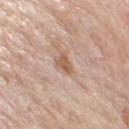The lesion was tiled from a total-body skin photograph and was not biopsied. Automated tile analysis of the lesion measured a mean CIELAB color near L≈57 a*≈20 b*≈30, roughly 10 lightness units darker than nearby skin, and a lesion-to-skin contrast of about 7.5 (normalized; higher = more distinct). The subject is a male roughly 75 years of age. This image is a 15 mm lesion crop taken from a total-body photograph. On the right upper arm.